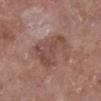notes: no biopsy performed (imaged during a skin exam)
anatomic site: the right lower leg
tile lighting: white-light illumination
image: ~15 mm crop, total-body skin-cancer survey
automated metrics: a lesion–skin lightness drop of about 8 and a normalized border contrast of about 6.5
lesion diameter: about 6 mm
subject: female, in their mid-70s